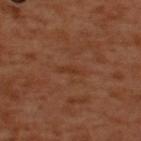Impression:
Imaged during a routine full-body skin examination; the lesion was not biopsied and no histopathology is available.
Context:
The subject is a male aged 48 to 52. This is a cross-polarized tile. Located on the upper back. A lesion tile, about 15 mm wide, cut from a 3D total-body photograph. Automated tile analysis of the lesion measured a lesion color around L≈35 a*≈23 b*≈31 in CIELAB and about 4 CIELAB-L* units darker than the surrounding skin.